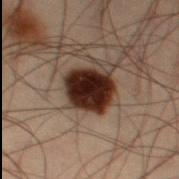notes = no biopsy performed (imaged during a skin exam); lighting = cross-polarized illumination; patient = male, approximately 55 years of age; site = the left thigh; image source = total-body-photography crop, ~15 mm field of view; lesion size = ~4.5 mm (longest diameter).